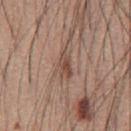Imaged during a routine full-body skin examination; the lesion was not biopsied and no histopathology is available. The lesion-visualizer software estimated an outline eccentricity of about 0.85 (0 = round, 1 = elongated) and two-axis asymmetry of about 0.3. It also reported lesion-presence confidence of about 100/100. This image is a 15 mm lesion crop taken from a total-body photograph. Captured under white-light illumination. The patient is a male roughly 60 years of age. The lesion is on the front of the torso.